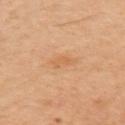Impression: No biopsy was performed on this lesion — it was imaged during a full skin examination and was not determined to be concerning. Image and clinical context: The lesion's longest dimension is about 3 mm. An algorithmic analysis of the crop reported a lesion color around L≈61 a*≈22 b*≈39 in CIELAB and a normalized lesion–skin contrast near 4.5. It also reported a border-irregularity index near 3/10, internal color variation of about 1.5 on a 0–10 scale, and peripheral color asymmetry of about 0.5. The software also gave an automated nevus-likeness rating near 0 out of 100 and a detector confidence of about 100 out of 100 that the crop contains a lesion. A male subject, roughly 40 years of age. Cropped from a total-body skin-imaging series; the visible field is about 15 mm. Captured under cross-polarized illumination. The lesion is on the left upper arm.